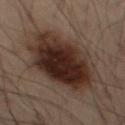Impression: The lesion was photographed on a routine skin check and not biopsied; there is no pathology result. Clinical summary: Captured under cross-polarized illumination. Longest diameter approximately 9.5 mm. A roughly 15 mm field-of-view crop from a total-body skin photograph. A male subject, approximately 55 years of age. The lesion is located on the left thigh.